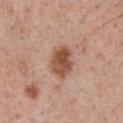No biopsy was performed on this lesion — it was imaged during a full skin examination and was not determined to be concerning. The lesion's longest dimension is about 4 mm. The lesion is located on the front of the torso. The lesion-visualizer software estimated a mean CIELAB color near L≈52 a*≈23 b*≈30, about 13 CIELAB-L* units darker than the surrounding skin, and a normalized border contrast of about 9.5. The software also gave a classifier nevus-likeness of about 90/100. Cropped from a total-body skin-imaging series; the visible field is about 15 mm. The subject is a male in their mid- to late 50s. Imaged with white-light lighting.